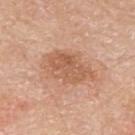biopsy status = catalogued during a skin exam; not biopsied | location = the upper back | size = ~6.5 mm (longest diameter) | acquisition = 15 mm crop, total-body photography | patient = male, aged around 80.